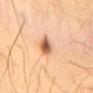{"biopsy_status": "not biopsied; imaged during a skin examination"}